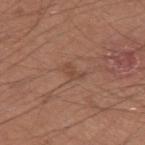  biopsy_status: not biopsied; imaged during a skin examination
  lighting: white-light
  automated_metrics:
    cielab_L: 46
    cielab_a: 20
    cielab_b: 28
    vs_skin_darker_L: 6.0
    vs_skin_contrast_norm: 5.0
  patient:
    sex: male
    age_approx: 35
  lesion_size:
    long_diameter_mm_approx: 2.5
  image:
    source: total-body photography crop
    field_of_view_mm: 15
  site: left upper arm